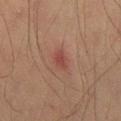follow-up — total-body-photography surveillance lesion; no biopsy
TBP lesion metrics — a footprint of about 3.5 mm² and a symmetry-axis asymmetry near 0.2; internal color variation of about 2.5 on a 0–10 scale and a peripheral color-asymmetry measure near 1; a classifier nevus-likeness of about 10/100 and a lesion-detection confidence of about 100/100
patient — male, aged around 35
size — about 2.5 mm
acquisition — ~15 mm crop, total-body skin-cancer survey
tile lighting — cross-polarized
site — the right thigh